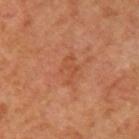Imaged during a routine full-body skin examination; the lesion was not biopsied and no histopathology is available.
The lesion is on the left upper arm.
The lesion-visualizer software estimated an area of roughly 4.5 mm², an eccentricity of roughly 0.85, and two-axis asymmetry of about 0.3. The analysis additionally found an automated nevus-likeness rating near 0 out of 100 and lesion-presence confidence of about 100/100.
This image is a 15 mm lesion crop taken from a total-body photograph.
This is a cross-polarized tile.
Approximately 3.5 mm at its widest.
A female subject approximately 65 years of age.